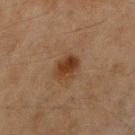Q: Was a biopsy performed?
A: imaged on a skin check; not biopsied
Q: Automated lesion metrics?
A: a footprint of about 6.5 mm² and an outline eccentricity of about 0.75 (0 = round, 1 = elongated); an average lesion color of about L≈33 a*≈18 b*≈29 (CIELAB), a lesion–skin lightness drop of about 9, and a lesion-to-skin contrast of about 9 (normalized; higher = more distinct); a border-irregularity index near 2/10 and peripheral color asymmetry of about 1.5
Q: Who is the patient?
A: female, about 70 years old
Q: Lesion location?
A: the right upper arm
Q: What is the imaging modality?
A: ~15 mm tile from a whole-body skin photo
Q: What lighting was used for the tile?
A: cross-polarized
Q: How large is the lesion?
A: ~3.5 mm (longest diameter)